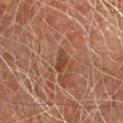Impression:
The lesion was tiled from a total-body skin photograph and was not biopsied.